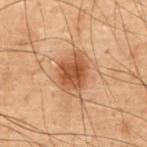Part of a total-body skin-imaging series; this lesion was reviewed on a skin check and was not flagged for biopsy. The lesion's longest dimension is about 5 mm. Located on the chest. Imaged with cross-polarized lighting. A lesion tile, about 15 mm wide, cut from a 3D total-body photograph. A male patient aged around 70. The total-body-photography lesion software estimated a footprint of about 12 mm², an eccentricity of roughly 0.7, and two-axis asymmetry of about 0.2.